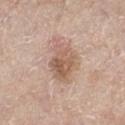Imaged during a routine full-body skin examination; the lesion was not biopsied and no histopathology is available.
Measured at roughly 5 mm in maximum diameter.
From the leg.
Captured under white-light illumination.
The patient is a female approximately 65 years of age.
A lesion tile, about 15 mm wide, cut from a 3D total-body photograph.
The total-body-photography lesion software estimated a mean CIELAB color near L≈59 a*≈18 b*≈28, a lesion–skin lightness drop of about 11, and a lesion-to-skin contrast of about 7 (normalized; higher = more distinct). And it measured a border-irregularity rating of about 3.5/10 and a color-variation rating of about 6/10. And it measured an automated nevus-likeness rating near 25 out of 100 and lesion-presence confidence of about 100/100.